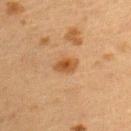Recorded during total-body skin imaging; not selected for excision or biopsy. The lesion is located on the upper back. An algorithmic analysis of the crop reported an average lesion color of about L≈44 a*≈20 b*≈35 (CIELAB), about 9 CIELAB-L* units darker than the surrounding skin, and a normalized lesion–skin contrast near 8. The software also gave a classifier nevus-likeness of about 95/100 and lesion-presence confidence of about 100/100. A female patient in their 40s. A 15 mm close-up tile from a total-body photography series done for melanoma screening. Captured under cross-polarized illumination.